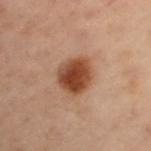Assessment: Part of a total-body skin-imaging series; this lesion was reviewed on a skin check and was not flagged for biopsy. Clinical summary: A female subject approximately 55 years of age. The lesion is on the left upper arm. A region of skin cropped from a whole-body photographic capture, roughly 15 mm wide. Approximately 4.5 mm at its widest.